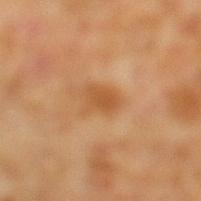location: the left lower leg | patient: male, approximately 60 years of age | image: total-body-photography crop, ~15 mm field of view | size: about 3.5 mm | TBP lesion metrics: a footprint of about 6 mm², an eccentricity of roughly 0.7, and a shape-asymmetry score of about 0.3 (0 = symmetric); border irregularity of about 3 on a 0–10 scale, a within-lesion color-variation index near 2/10, and radial color variation of about 0.5; a lesion-detection confidence of about 100/100.